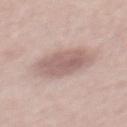<case>
  <biopsy_status>not biopsied; imaged during a skin examination</biopsy_status>
  <site>mid back</site>
  <image>
    <source>total-body photography crop</source>
    <field_of_view_mm>15</field_of_view_mm>
  </image>
  <patient>
    <sex>male</sex>
    <age_approx>55</age_approx>
  </patient>
</case>